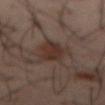{
  "biopsy_status": "not biopsied; imaged during a skin examination",
  "lighting": "cross-polarized",
  "patient": {
    "sex": "male",
    "age_approx": 40
  },
  "site": "abdomen",
  "image": {
    "source": "total-body photography crop",
    "field_of_view_mm": 15
  }
}